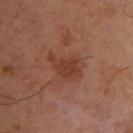Q: Was a biopsy performed?
A: total-body-photography surveillance lesion; no biopsy
Q: Patient demographics?
A: male, aged around 50
Q: What lighting was used for the tile?
A: cross-polarized illumination
Q: What is the imaging modality?
A: total-body-photography crop, ~15 mm field of view
Q: Lesion location?
A: the upper back